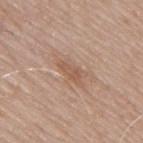This lesion was catalogued during total-body skin photography and was not selected for biopsy.
The total-body-photography lesion software estimated a footprint of about 3 mm², an eccentricity of roughly 0.9, and a symmetry-axis asymmetry near 0.3. The analysis additionally found border irregularity of about 3.5 on a 0–10 scale and radial color variation of about 0.
Approximately 3 mm at its widest.
From the mid back.
This image is a 15 mm lesion crop taken from a total-body photograph.
The patient is a male approximately 65 years of age.
The tile uses white-light illumination.